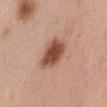– follow-up: catalogued during a skin exam; not biopsied
– image: 15 mm crop, total-body photography
– subject: female, about 50 years old
– body site: the abdomen